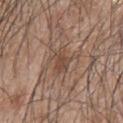Imaged during a routine full-body skin examination; the lesion was not biopsied and no histopathology is available. The total-body-photography lesion software estimated a lesion area of about 5 mm², an eccentricity of roughly 0.8, and two-axis asymmetry of about 0.3. The software also gave a mean CIELAB color near L≈46 a*≈17 b*≈27. The analysis additionally found border irregularity of about 3.5 on a 0–10 scale and peripheral color asymmetry of about 0.5. The software also gave a nevus-likeness score of about 0/100 and a lesion-detection confidence of about 70/100. Captured under white-light illumination. A lesion tile, about 15 mm wide, cut from a 3D total-body photograph. From the upper back. The subject is a male aged 43 to 47.